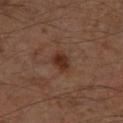<record>
  <biopsy_status>not biopsied; imaged during a skin examination</biopsy_status>
  <lesion_size>
    <long_diameter_mm_approx>2.5</long_diameter_mm_approx>
  </lesion_size>
  <patient>
    <sex>male</sex>
    <age_approx>60</age_approx>
  </patient>
  <site>leg</site>
  <image>
    <source>total-body photography crop</source>
    <field_of_view_mm>15</field_of_view_mm>
  </image>
  <automated_metrics>
    <vs_skin_darker_L>9.0</vs_skin_darker_L>
    <border_irregularity_0_10>2.0</border_irregularity_0_10>
    <color_variation_0_10>2.0</color_variation_0_10>
    <nevus_likeness_0_100>90</nevus_likeness_0_100>
    <lesion_detection_confidence_0_100>100</lesion_detection_confidence_0_100>
  </automated_metrics>
</record>